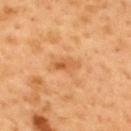Clinical impression: The lesion was tiled from a total-body skin photograph and was not biopsied. Clinical summary: An algorithmic analysis of the crop reported an average lesion color of about L≈59 a*≈26 b*≈44 (CIELAB), about 9 CIELAB-L* units darker than the surrounding skin, and a normalized border contrast of about 6. The analysis additionally found an automated nevus-likeness rating near 0 out of 100. On the mid back. Imaged with cross-polarized lighting. A region of skin cropped from a whole-body photographic capture, roughly 15 mm wide. Approximately 3.5 mm at its widest. A male patient aged approximately 50.